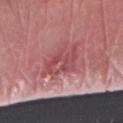notes — total-body-photography surveillance lesion; no biopsy
lesion size — ~5 mm (longest diameter)
subject — male, about 80 years old
acquisition — 15 mm crop, total-body photography
body site — the right forearm
lighting — white-light